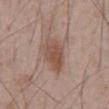Imaged during a routine full-body skin examination; the lesion was not biopsied and no histopathology is available. Located on the chest. The patient is a male aged 63 to 67. Captured under white-light illumination. A close-up tile cropped from a whole-body skin photograph, about 15 mm across.